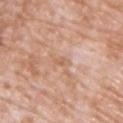The lesion was photographed on a routine skin check and not biopsied; there is no pathology result. Cropped from a whole-body photographic skin survey; the tile spans about 15 mm. The lesion is on the chest. Captured under white-light illumination. A male patient roughly 70 years of age.